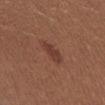The lesion was tiled from a total-body skin photograph and was not biopsied.
The subject is a female approximately 25 years of age.
The total-body-photography lesion software estimated a footprint of about 4.5 mm², a shape eccentricity near 0.85, and a symmetry-axis asymmetry near 0.25. It also reported about 8 CIELAB-L* units darker than the surrounding skin and a lesion-to-skin contrast of about 7 (normalized; higher = more distinct). The analysis additionally found a within-lesion color-variation index near 1.5/10.
Longest diameter approximately 3 mm.
On the abdomen.
A roughly 15 mm field-of-view crop from a total-body skin photograph.